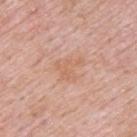biopsy_status: not biopsied; imaged during a skin examination
automated_metrics:
  border_irregularity_0_10: 5.5
  color_variation_0_10: 2.0
  peripheral_color_asymmetry: 0.5
  nevus_likeness_0_100: 0
  lesion_detection_confidence_0_100: 100
patient:
  sex: male
  age_approx: 55
lesion_size:
  long_diameter_mm_approx: 3.5
lighting: white-light
site: upper back
image:
  source: total-body photography crop
  field_of_view_mm: 15